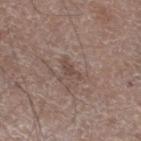Q: Was a biopsy performed?
A: imaged on a skin check; not biopsied
Q: Where on the body is the lesion?
A: the left lower leg
Q: Who is the patient?
A: male, aged 58–62
Q: Automated lesion metrics?
A: a nevus-likeness score of about 0/100
Q: How was this image acquired?
A: ~15 mm crop, total-body skin-cancer survey
Q: Illumination type?
A: white-light
Q: What is the lesion's diameter?
A: about 3 mm